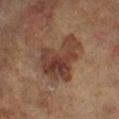{
  "biopsy_status": "not biopsied; imaged during a skin examination",
  "site": "left lower leg",
  "image": {
    "source": "total-body photography crop",
    "field_of_view_mm": 15
  },
  "automated_metrics": {
    "border_irregularity_0_10": 5.5,
    "color_variation_0_10": 6.5,
    "peripheral_color_asymmetry": 2.0,
    "nevus_likeness_0_100": 40,
    "lesion_detection_confidence_0_100": 100
  },
  "lighting": "cross-polarized",
  "patient": {
    "sex": "female",
    "age_approx": 75
  }
}